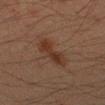Notes:
– workup · imaged on a skin check; not biopsied
– image source · total-body-photography crop, ~15 mm field of view
– subject · male, about 35 years old
– tile lighting · cross-polarized
– size · ~5 mm (longest diameter)
– body site · the arm